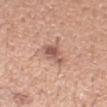Imaged with white-light lighting. The total-body-photography lesion software estimated a lesion area of about 6 mm², a shape eccentricity near 0.6, and a shape-asymmetry score of about 0.4 (0 = symmetric). The software also gave a lesion color around L≈57 a*≈22 b*≈26 in CIELAB, a lesion–skin lightness drop of about 11, and a normalized border contrast of about 7.5. And it measured a lesion-detection confidence of about 100/100. About 3 mm across. The patient is a male aged 38–42. On the arm. A roughly 15 mm field-of-view crop from a total-body skin photograph.